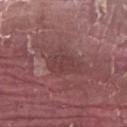No biopsy was performed on this lesion — it was imaged during a full skin examination and was not determined to be concerning. A lesion tile, about 15 mm wide, cut from a 3D total-body photograph. A male patient, aged 38–42. Located on the left forearm.